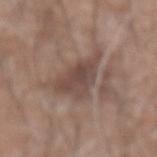  biopsy_status: not biopsied; imaged during a skin examination
  site: back
  image:
    source: total-body photography crop
    field_of_view_mm: 15
  automated_metrics:
    cielab_L: 46
    cielab_a: 17
    cielab_b: 22
    vs_skin_darker_L: 9.0
    vs_skin_contrast_norm: 7.5
  lighting: white-light
  patient:
    sex: male
    age_approx: 60
  lesion_size:
    long_diameter_mm_approx: 5.0